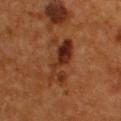Impression:
Part of a total-body skin-imaging series; this lesion was reviewed on a skin check and was not flagged for biopsy.
Background:
A 15 mm close-up extracted from a 3D total-body photography capture. The lesion-visualizer software estimated a shape-asymmetry score of about 0.4 (0 = symmetric). And it measured a mean CIELAB color near L≈30 a*≈22 b*≈30, roughly 9 lightness units darker than nearby skin, and a lesion-to-skin contrast of about 9 (normalized; higher = more distinct). It also reported a border-irregularity index near 6.5/10, a color-variation rating of about 9/10, and a peripheral color-asymmetry measure near 3.5. A male patient in their mid-50s. Approximately 5.5 mm at its widest. This is a cross-polarized tile. From the upper back.